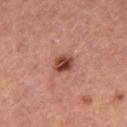follow-up: catalogued during a skin exam; not biopsied
diameter: ≈2.5 mm
image source: ~15 mm crop, total-body skin-cancer survey
lighting: cross-polarized illumination
subject: female, in their mid- to late 40s
automated lesion analysis: an area of roughly 4.5 mm², an outline eccentricity of about 0.6 (0 = round, 1 = elongated), and a symmetry-axis asymmetry near 0.2; a border-irregularity rating of about 2/10, a within-lesion color-variation index near 7/10, and radial color variation of about 2.5; a classifier nevus-likeness of about 95/100 and lesion-presence confidence of about 100/100
anatomic site: the leg